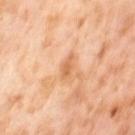Findings:
* workup: total-body-photography surveillance lesion; no biopsy
* tile lighting: cross-polarized
* patient: female, approximately 55 years of age
* lesion size: ≈3 mm
* location: the leg
* image: 15 mm crop, total-body photography